Cropped from a whole-body photographic skin survey; the tile spans about 15 mm. The recorded lesion diameter is about 1 mm. The lesion-visualizer software estimated a lesion-to-skin contrast of about 3.5 (normalized; higher = more distinct). The software also gave border irregularity of about 3 on a 0–10 scale and radial color variation of about 0. A male subject, aged around 65. Located on the chest. Captured under white-light illumination — 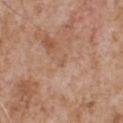biopsy diagnosis: a superficial basal cell carcinoma — a skin cancer.